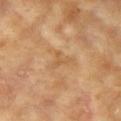biopsy_status: not biopsied; imaged during a skin examination
patient:
  sex: female
  age_approx: 75
lesion_size:
  long_diameter_mm_approx: 2.5
image:
  source: total-body photography crop
  field_of_view_mm: 15
automated_metrics:
  area_mm2_approx: 3.0
  eccentricity: 0.55
site: left upper arm
lighting: cross-polarized